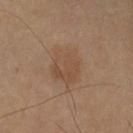The lesion was tiled from a total-body skin photograph and was not biopsied. On the arm. A male subject about 70 years old. Cropped from a total-body skin-imaging series; the visible field is about 15 mm.